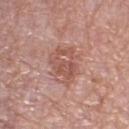Captured during whole-body skin photography for melanoma surveillance; the lesion was not biopsied.
The lesion-visualizer software estimated roughly 9 lightness units darker than nearby skin. The analysis additionally found internal color variation of about 4.5 on a 0–10 scale and a peripheral color-asymmetry measure near 1.5. And it measured lesion-presence confidence of about 100/100.
Imaged with white-light lighting.
On the right lower leg.
Measured at roughly 5 mm in maximum diameter.
Cropped from a whole-body photographic skin survey; the tile spans about 15 mm.
A female patient, in their 70s.